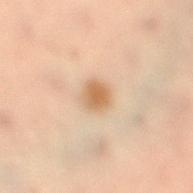Acquisition and patient details:
The tile uses cross-polarized illumination. A close-up tile cropped from a whole-body skin photograph, about 15 mm across. From the left leg. The subject is a female approximately 55 years of age. The total-body-photography lesion software estimated a lesion area of about 5.5 mm² and a symmetry-axis asymmetry near 0.15. The analysis additionally found a mean CIELAB color near L≈54 a*≈16 b*≈32, roughly 9 lightness units darker than nearby skin, and a normalized border contrast of about 7.5. And it measured a border-irregularity index near 1.5/10 and radial color variation of about 1. The software also gave a nevus-likeness score of about 95/100.